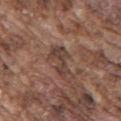notes = imaged on a skin check; not biopsied
size = ≈4 mm
anatomic site = the right upper arm
acquisition = ~15 mm tile from a whole-body skin photo
subject = male, aged 73–77
tile lighting = white-light